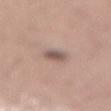Part of a total-body skin-imaging series; this lesion was reviewed on a skin check and was not flagged for biopsy. Cropped from a whole-body photographic skin survey; the tile spans about 15 mm. Longest diameter approximately 2.5 mm. The subject is a male aged 43–47. This is a white-light tile. Located on the lower back.